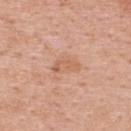Findings:
– biopsy status · no biopsy performed (imaged during a skin exam)
– tile lighting · white-light illumination
– lesion diameter · about 3 mm
– subject · female, aged 48–52
– imaging modality · ~15 mm tile from a whole-body skin photo
– site · the upper back